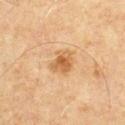{
  "biopsy_status": "not biopsied; imaged during a skin examination",
  "lesion_size": {
    "long_diameter_mm_approx": 3.0
  },
  "site": "chest",
  "image": {
    "source": "total-body photography crop",
    "field_of_view_mm": 15
  },
  "patient": {
    "sex": "male",
    "age_approx": 65
  },
  "lighting": "cross-polarized"
}